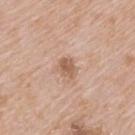biopsy status: no biopsy performed (imaged during a skin exam); patient: male, aged 63 to 67; imaging modality: 15 mm crop, total-body photography; anatomic site: the upper back; size: ≈2.5 mm.